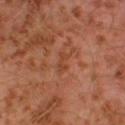Recorded during total-body skin imaging; not selected for excision or biopsy. Measured at roughly 2.5 mm in maximum diameter. The subject is a male aged 28–32. The tile uses cross-polarized illumination. Cropped from a total-body skin-imaging series; the visible field is about 15 mm. The lesion-visualizer software estimated an area of roughly 3 mm², an eccentricity of roughly 0.9, and a shape-asymmetry score of about 0.4 (0 = symmetric). The analysis additionally found border irregularity of about 4.5 on a 0–10 scale, a within-lesion color-variation index near 0/10, and peripheral color asymmetry of about 0. Located on the left leg.